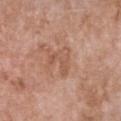| key | value |
|---|---|
| workup | imaged on a skin check; not biopsied |
| imaging modality | ~15 mm tile from a whole-body skin photo |
| TBP lesion metrics | a lesion area of about 7 mm², a shape eccentricity near 0.35, and a shape-asymmetry score of about 0.6 (0 = symmetric); an automated nevus-likeness rating near 0 out of 100 and a detector confidence of about 100 out of 100 that the crop contains a lesion |
| size | about 3.5 mm |
| body site | the chest |
| illumination | white-light |
| patient | female, approximately 75 years of age |